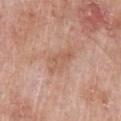Q: Was this lesion biopsied?
A: imaged on a skin check; not biopsied
Q: Lesion location?
A: the chest
Q: Automated lesion metrics?
A: a mean CIELAB color near L≈58 a*≈21 b*≈30, about 7 CIELAB-L* units darker than the surrounding skin, and a lesion-to-skin contrast of about 5 (normalized; higher = more distinct); an automated nevus-likeness rating near 0 out of 100 and a detector confidence of about 100 out of 100 that the crop contains a lesion
Q: What lighting was used for the tile?
A: white-light illumination
Q: What is the lesion's diameter?
A: about 4 mm
Q: Patient demographics?
A: male, about 65 years old
Q: What is the imaging modality?
A: ~15 mm tile from a whole-body skin photo